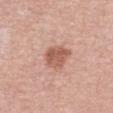biopsy status = total-body-photography surveillance lesion; no biopsy
patient = male, aged 53–57
imaging modality = 15 mm crop, total-body photography
site = the chest